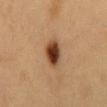Assessment: No biopsy was performed on this lesion — it was imaged during a full skin examination and was not determined to be concerning. Context: On the front of the torso. A 15 mm close-up tile from a total-body photography series done for melanoma screening. A male subject, in their 60s.